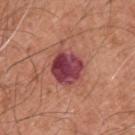<tbp_lesion>
<biopsy_status>not biopsied; imaged during a skin examination</biopsy_status>
<site>upper back</site>
<lighting>white-light</lighting>
<lesion_size>
  <long_diameter_mm_approx>4.0</long_diameter_mm_approx>
</lesion_size>
<automated_metrics>
  <area_mm2_approx>13.0</area_mm2_approx>
  <eccentricity>0.35</eccentricity>
  <border_irregularity_0_10>1.5</border_irregularity_0_10>
  <color_variation_0_10>7.5</color_variation_0_10>
</automated_metrics>
<image>
  <source>total-body photography crop</source>
  <field_of_view_mm>15</field_of_view_mm>
</image>
<patient>
  <sex>male</sex>
  <age_approx>60</age_approx>
</patient>
</tbp_lesion>